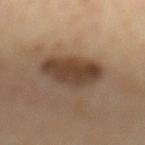follow-up: total-body-photography surveillance lesion; no biopsy | lighting: cross-polarized | image: ~15 mm crop, total-body skin-cancer survey | image-analysis metrics: an area of roughly 17 mm², an eccentricity of roughly 0.6, and two-axis asymmetry of about 0.15; a detector confidence of about 100 out of 100 that the crop contains a lesion | body site: the mid back | patient: male, aged approximately 65 | diameter: ~5 mm (longest diameter).